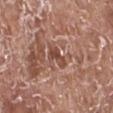lesion diameter=~3 mm (longest diameter); imaging modality=total-body-photography crop, ~15 mm field of view; lighting=white-light illumination; automated metrics=a nevus-likeness score of about 0/100 and lesion-presence confidence of about 95/100; subject=male, roughly 75 years of age; anatomic site=the right lower leg.